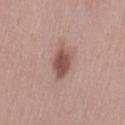{"biopsy_status": "not biopsied; imaged during a skin examination", "site": "left thigh", "image": {"source": "total-body photography crop", "field_of_view_mm": 15}, "patient": {"sex": "female", "age_approx": 40}, "automated_metrics": {"eccentricity": 0.75, "cielab_L": 51, "cielab_a": 20, "cielab_b": 24, "vs_skin_darker_L": 13.0, "vs_skin_contrast_norm": 9.0}, "lesion_size": {"long_diameter_mm_approx": 4.0}}